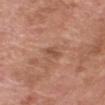Assessment: The lesion was tiled from a total-body skin photograph and was not biopsied. Background: A lesion tile, about 15 mm wide, cut from a 3D total-body photograph. Imaged with white-light lighting. About 2.5 mm across. A male patient, about 60 years old. Located on the head or neck. The total-body-photography lesion software estimated a mean CIELAB color near L≈50 a*≈23 b*≈30, a lesion–skin lightness drop of about 8, and a normalized lesion–skin contrast near 6. The analysis additionally found a border-irregularity rating of about 3/10. The software also gave a classifier nevus-likeness of about 0/100 and lesion-presence confidence of about 100/100.